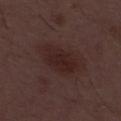Captured during whole-body skin photography for melanoma surveillance; the lesion was not biopsied. Automated image analysis of the tile measured a lesion color around L≈23 a*≈17 b*≈17 in CIELAB, a lesion–skin lightness drop of about 6, and a normalized lesion–skin contrast near 7. And it measured a nevus-likeness score of about 25/100 and a lesion-detection confidence of about 100/100. The lesion's longest dimension is about 5.5 mm. A male subject, aged 48–52. This is a white-light tile. A 15 mm crop from a total-body photograph taken for skin-cancer surveillance.